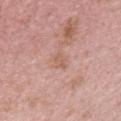<record>
  <biopsy_status>not biopsied; imaged during a skin examination</biopsy_status>
  <image>
    <source>total-body photography crop</source>
    <field_of_view_mm>15</field_of_view_mm>
  </image>
  <automated_metrics>
    <area_mm2_approx>2.5</area_mm2_approx>
    <shape_asymmetry>0.35</shape_asymmetry>
    <nevus_likeness_0_100>0</nevus_likeness_0_100>
    <lesion_detection_confidence_0_100>100</lesion_detection_confidence_0_100>
  </automated_metrics>
  <patient>
    <sex>male</sex>
    <age_approx>75</age_approx>
  </patient>
  <site>head or neck</site>
  <lesion_size>
    <long_diameter_mm_approx>2.5</long_diameter_mm_approx>
  </lesion_size>
  <lighting>white-light</lighting>
</record>